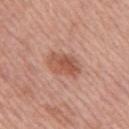notes — catalogued during a skin exam; not biopsied | automated metrics — a lesion area of about 9 mm², an eccentricity of roughly 0.8, and a symmetry-axis asymmetry near 0.15; an average lesion color of about L≈54 a*≈25 b*≈30 (CIELAB) and about 10 CIELAB-L* units darker than the surrounding skin | acquisition — ~15 mm tile from a whole-body skin photo | location — the right upper arm | lesion size — ~4 mm (longest diameter) | tile lighting — white-light illumination | patient — male, approximately 60 years of age.